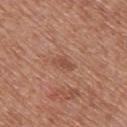Captured under white-light illumination. A region of skin cropped from a whole-body photographic capture, roughly 15 mm wide. The total-body-photography lesion software estimated an area of roughly 3 mm², a shape eccentricity near 0.85, and a symmetry-axis asymmetry near 0.35. It also reported a within-lesion color-variation index near 0/10. The analysis additionally found a nevus-likeness score of about 5/100 and a detector confidence of about 100 out of 100 that the crop contains a lesion. A male subject aged 63 to 67. On the upper back.